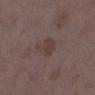Part of a total-body skin-imaging series; this lesion was reviewed on a skin check and was not flagged for biopsy.
The lesion is on the left lower leg.
A female patient aged 33–37.
The recorded lesion diameter is about 3 mm.
Captured under white-light illumination.
A 15 mm crop from a total-body photograph taken for skin-cancer surveillance.